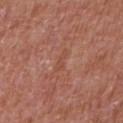{"biopsy_status": "not biopsied; imaged during a skin examination", "image": {"source": "total-body photography crop", "field_of_view_mm": 15}, "site": "front of the torso", "patient": {"sex": "male", "age_approx": 65}}